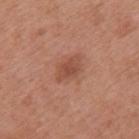Q: Was a biopsy performed?
A: catalogued during a skin exam; not biopsied
Q: Illumination type?
A: white-light
Q: What are the patient's age and sex?
A: male, aged 68 to 72
Q: Automated lesion metrics?
A: a lesion color around L≈49 a*≈25 b*≈30 in CIELAB, a lesion–skin lightness drop of about 9, and a lesion-to-skin contrast of about 6.5 (normalized; higher = more distinct); internal color variation of about 2.5 on a 0–10 scale and peripheral color asymmetry of about 0.5; an automated nevus-likeness rating near 70 out of 100 and a lesion-detection confidence of about 100/100
Q: Lesion location?
A: the mid back
Q: What is the lesion's diameter?
A: ≈3.5 mm
Q: What is the imaging modality?
A: total-body-photography crop, ~15 mm field of view